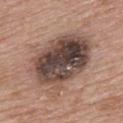Notes:
– workup · imaged on a skin check; not biopsied
– patient · female, in their 50s
– body site · the upper back
– illumination · white-light
– image · ~15 mm crop, total-body skin-cancer survey
– lesion size · about 8 mm